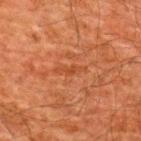biopsy status = no biopsy performed (imaged during a skin exam)
subject = male, about 60 years old
lighting = cross-polarized illumination
image = ~15 mm tile from a whole-body skin photo
diameter = ~3.5 mm (longest diameter)
site = the upper back